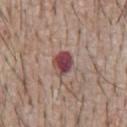Captured during whole-body skin photography for melanoma surveillance; the lesion was not biopsied.
The lesion is on the chest.
A male subject in their mid- to late 60s.
Cropped from a total-body skin-imaging series; the visible field is about 15 mm.
Approximately 3 mm at its widest.